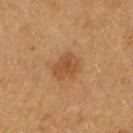biopsy status: catalogued during a skin exam; not biopsied | imaging modality: ~15 mm tile from a whole-body skin photo | body site: the right forearm | subject: female, aged 38–42 | size: ~3.5 mm (longest diameter) | tile lighting: cross-polarized illumination.